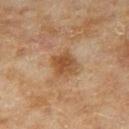Notes:
– patient — female, in their 60s
– imaging modality — total-body-photography crop, ~15 mm field of view
– diameter — ≈3 mm
– site — the leg